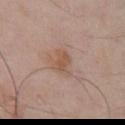biopsy status=imaged on a skin check; not biopsied | site=the front of the torso | subject=male, aged approximately 55 | illumination=white-light illumination | acquisition=~15 mm tile from a whole-body skin photo | lesion diameter=≈3 mm.